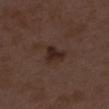biopsy status: no biopsy performed (imaged during a skin exam)
patient: female, approximately 40 years of age
lesion diameter: ≈3 mm
location: the left upper arm
tile lighting: white-light illumination
imaging modality: ~15 mm crop, total-body skin-cancer survey